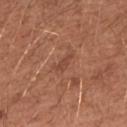Q: Is there a histopathology result?
A: no biopsy performed (imaged during a skin exam)
Q: Patient demographics?
A: female, aged 38–42
Q: Automated lesion metrics?
A: a symmetry-axis asymmetry near 0.5; border irregularity of about 5.5 on a 0–10 scale and a color-variation rating of about 0/10; a nevus-likeness score of about 0/100 and lesion-presence confidence of about 95/100
Q: What kind of image is this?
A: ~15 mm crop, total-body skin-cancer survey
Q: Lesion location?
A: the left forearm
Q: What lighting was used for the tile?
A: white-light
Q: What is the lesion's diameter?
A: ~3 mm (longest diameter)